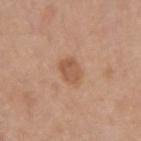Q: Was a biopsy performed?
A: total-body-photography surveillance lesion; no biopsy
Q: What is the anatomic site?
A: the left forearm
Q: Who is the patient?
A: female, in their mid- to late 30s
Q: What kind of image is this?
A: ~15 mm crop, total-body skin-cancer survey